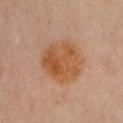Q: Was this lesion biopsied?
A: catalogued during a skin exam; not biopsied
Q: What is the imaging modality?
A: 15 mm crop, total-body photography
Q: How was the tile lit?
A: cross-polarized
Q: What did automated image analysis measure?
A: a lesion area of about 21 mm², an outline eccentricity of about 0.45 (0 = round, 1 = elongated), and a symmetry-axis asymmetry near 0.15; a classifier nevus-likeness of about 75/100 and a lesion-detection confidence of about 100/100
Q: Where on the body is the lesion?
A: the chest
Q: What are the patient's age and sex?
A: female, aged 58–62
Q: Lesion size?
A: ~5.5 mm (longest diameter)